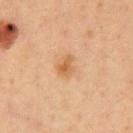| feature | finding |
|---|---|
| notes | total-body-photography surveillance lesion; no biopsy |
| patient | male, about 50 years old |
| acquisition | total-body-photography crop, ~15 mm field of view |
| illumination | cross-polarized illumination |
| location | the chest |